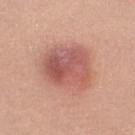Impression: Captured during whole-body skin photography for melanoma surveillance; the lesion was not biopsied. Background: This is a white-light tile. A lesion tile, about 15 mm wide, cut from a 3D total-body photograph. The lesion is located on the left lower leg. A female patient aged 33–37. Longest diameter approximately 6 mm.